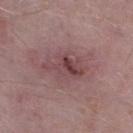The lesion was photographed on a routine skin check and not biopsied; there is no pathology result. A 15 mm close-up tile from a total-body photography series done for melanoma screening. The recorded lesion diameter is about 5 mm. The patient is a male in their mid-70s. From the right lower leg. The total-body-photography lesion software estimated a lesion area of about 9 mm² and two-axis asymmetry of about 0.4. The software also gave an average lesion color of about L≈44 a*≈22 b*≈17 (CIELAB), roughly 9 lightness units darker than nearby skin, and a normalized border contrast of about 7. It also reported a border-irregularity rating of about 5.5/10 and a peripheral color-asymmetry measure near 3. The tile uses white-light illumination.